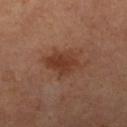This lesion was catalogued during total-body skin photography and was not selected for biopsy. A roughly 15 mm field-of-view crop from a total-body skin photograph. The patient is a female aged around 65. The tile uses cross-polarized illumination. Longest diameter approximately 4.5 mm. Located on the right lower leg.